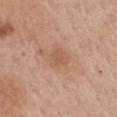Background: The lesion-visualizer software estimated a footprint of about 4.5 mm² and an eccentricity of roughly 0.65. It also reported an average lesion color of about L≈56 a*≈21 b*≈32 (CIELAB) and a normalized border contrast of about 5. And it measured a nevus-likeness score of about 0/100. A roughly 15 mm field-of-view crop from a total-body skin photograph. Located on the mid back. Imaged with white-light lighting. The subject is a female in their mid- to late 60s. Approximately 2.5 mm at its widest.